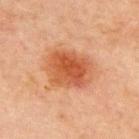notes: no biopsy performed (imaged during a skin exam) | automated lesion analysis: an average lesion color of about L≈46 a*≈25 b*≈33 (CIELAB), a lesion–skin lightness drop of about 10, and a lesion-to-skin contrast of about 8 (normalized; higher = more distinct); a border-irregularity rating of about 1.5/10 and a color-variation rating of about 5/10 | subject: male, aged approximately 70 | acquisition: total-body-photography crop, ~15 mm field of view | location: the chest | tile lighting: cross-polarized illumination | lesion diameter: about 5.5 mm.